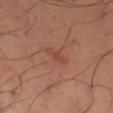No biopsy was performed on this lesion — it was imaged during a full skin examination and was not determined to be concerning. A male subject roughly 40 years of age. Located on the left thigh. The total-body-photography lesion software estimated roughly 6 lightness units darker than nearby skin and a normalized border contrast of about 5. A 15 mm close-up tile from a total-body photography series done for melanoma screening. About 3 mm across.